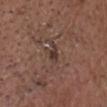workup = imaged on a skin check; not biopsied
subject = male, aged 73–77
location = the chest
acquisition = ~15 mm tile from a whole-body skin photo